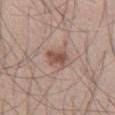• follow-up — no biopsy performed (imaged during a skin exam)
• lesion size — about 2.5 mm
• subject — male, aged approximately 30
• lighting — white-light illumination
• image source — ~15 mm tile from a whole-body skin photo
• anatomic site — the right thigh